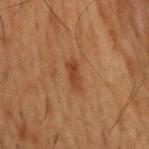Assessment:
No biopsy was performed on this lesion — it was imaged during a full skin examination and was not determined to be concerning.
Image and clinical context:
A male subject, aged around 55. About 3.5 mm across. Captured under cross-polarized illumination. A 15 mm crop from a total-body photograph taken for skin-cancer surveillance. Located on the upper back.